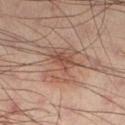follow-up=no biopsy performed (imaged during a skin exam) | image source=15 mm crop, total-body photography | anatomic site=the left lower leg | patient=male, roughly 40 years of age.